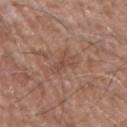Assessment:
Part of a total-body skin-imaging series; this lesion was reviewed on a skin check and was not flagged for biopsy.
Context:
The patient is a male aged around 75. A roughly 15 mm field-of-view crop from a total-body skin photograph. Imaged with white-light lighting. The lesion's longest dimension is about 4 mm. From the right upper arm.